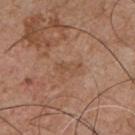The lesion was photographed on a routine skin check and not biopsied; there is no pathology result.
A male subject aged 53 to 57.
The lesion's longest dimension is about 3 mm.
On the chest.
The total-body-photography lesion software estimated a lesion area of about 3 mm² and a shape-asymmetry score of about 0.35 (0 = symmetric).
A 15 mm crop from a total-body photograph taken for skin-cancer surveillance.